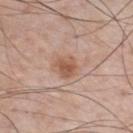Assessment:
The lesion was photographed on a routine skin check and not biopsied; there is no pathology result.
Background:
Measured at roughly 2.5 mm in maximum diameter. A male patient aged 48 to 52. This is a white-light tile. Cropped from a total-body skin-imaging series; the visible field is about 15 mm. On the front of the torso.